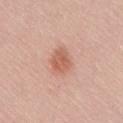The lesion was photographed on a routine skin check and not biopsied; there is no pathology result. The lesion is on the lower back. A male patient roughly 80 years of age. Cropped from a whole-body photographic skin survey; the tile spans about 15 mm. Approximately 3.5 mm at its widest.